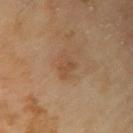Impression:
Captured during whole-body skin photography for melanoma surveillance; the lesion was not biopsied.
Acquisition and patient details:
The patient is a male about 65 years old. Imaged with cross-polarized lighting. The lesion's longest dimension is about 2.5 mm. Cropped from a total-body skin-imaging series; the visible field is about 15 mm. The lesion is on the right upper arm.